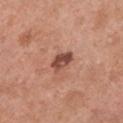Part of a total-body skin-imaging series; this lesion was reviewed on a skin check and was not flagged for biopsy. The subject is a female aged approximately 50. From the chest. A close-up tile cropped from a whole-body skin photograph, about 15 mm across. The tile uses white-light illumination.